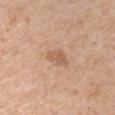Q: Was this lesion biopsied?
A: total-body-photography surveillance lesion; no biopsy
Q: What lighting was used for the tile?
A: white-light illumination
Q: What is the imaging modality?
A: ~15 mm crop, total-body skin-cancer survey
Q: What is the anatomic site?
A: the right upper arm
Q: How large is the lesion?
A: about 3 mm
Q: What are the patient's age and sex?
A: male, aged 73 to 77